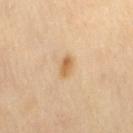Part of a total-body skin-imaging series; this lesion was reviewed on a skin check and was not flagged for biopsy. Automated image analysis of the tile measured a footprint of about 3.5 mm², a shape eccentricity near 0.75, and a shape-asymmetry score of about 0.2 (0 = symmetric). And it measured a lesion color around L≈65 a*≈18 b*≈41 in CIELAB and a normalized lesion–skin contrast near 7.5. And it measured border irregularity of about 1.5 on a 0–10 scale, a color-variation rating of about 3.5/10, and radial color variation of about 1. The lesion is located on the leg. The subject is a female aged 68–72. This image is a 15 mm lesion crop taken from a total-body photograph. The lesion's longest dimension is about 2.5 mm. Captured under cross-polarized illumination.